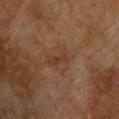<tbp_lesion>
  <biopsy_status>not biopsied; imaged during a skin examination</biopsy_status>
  <patient>
    <sex>male</sex>
    <age_approx>75</age_approx>
  </patient>
  <image>
    <source>total-body photography crop</source>
    <field_of_view_mm>15</field_of_view_mm>
  </image>
  <lesion_size>
    <long_diameter_mm_approx>3.0</long_diameter_mm_approx>
  </lesion_size>
  <lighting>cross-polarized</lighting>
  <site>upper back</site>
</tbp_lesion>